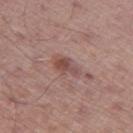Q: Was this lesion biopsied?
A: imaged on a skin check; not biopsied
Q: Patient demographics?
A: male, aged around 65
Q: What is the imaging modality?
A: ~15 mm crop, total-body skin-cancer survey
Q: Where on the body is the lesion?
A: the left thigh